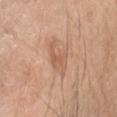| feature | finding |
|---|---|
| follow-up | catalogued during a skin exam; not biopsied |
| illumination | white-light illumination |
| lesion size | ~4 mm (longest diameter) |
| automated metrics | an area of roughly 6.5 mm² and a shape-asymmetry score of about 0.45 (0 = symmetric); border irregularity of about 6.5 on a 0–10 scale and radial color variation of about 0.5 |
| acquisition | 15 mm crop, total-body photography |
| subject | male, aged approximately 50 |
| body site | the head or neck |